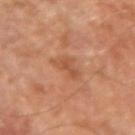| key | value |
|---|---|
| biopsy status | no biopsy performed (imaged during a skin exam) |
| patient | male, about 70 years old |
| body site | the right upper arm |
| size | ≈3.5 mm |
| image source | 15 mm crop, total-body photography |
| lighting | cross-polarized illumination |